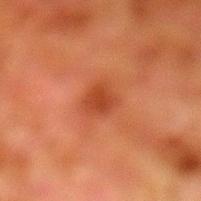Impression: The lesion was tiled from a total-body skin photograph and was not biopsied. Context: The lesion is on the left lower leg. A male subject, aged around 80. The recorded lesion diameter is about 2.5 mm. Captured under cross-polarized illumination. Cropped from a whole-body photographic skin survey; the tile spans about 15 mm. The total-body-photography lesion software estimated a border-irregularity index near 3/10, a color-variation rating of about 1.5/10, and a peripheral color-asymmetry measure near 0.5. It also reported a classifier nevus-likeness of about 25/100 and a detector confidence of about 100 out of 100 that the crop contains a lesion.